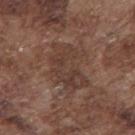Impression:
This lesion was catalogued during total-body skin photography and was not selected for biopsy.
Image and clinical context:
A close-up tile cropped from a whole-body skin photograph, about 15 mm across. A male patient roughly 75 years of age. The lesion is located on the upper back.